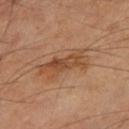The lesion was photographed on a routine skin check and not biopsied; there is no pathology result. On the leg. This image is a 15 mm lesion crop taken from a total-body photograph. The patient is a male approximately 65 years of age. The recorded lesion diameter is about 5.5 mm.